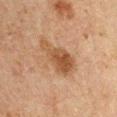A lesion tile, about 15 mm wide, cut from a 3D total-body photograph. Located on the left upper arm. An algorithmic analysis of the crop reported two-axis asymmetry of about 0.4. And it measured a nevus-likeness score of about 60/100 and a detector confidence of about 100 out of 100 that the crop contains a lesion. Imaged with cross-polarized lighting. The patient is a male aged approximately 50.